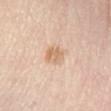Impression: The lesion was tiled from a total-body skin photograph and was not biopsied. Acquisition and patient details: The lesion is on the leg. The patient is a female in their 40s. Captured under cross-polarized illumination. The lesion-visualizer software estimated an area of roughly 6 mm² and an outline eccentricity of about 0.6 (0 = round, 1 = elongated). And it measured a classifier nevus-likeness of about 15/100 and a lesion-detection confidence of about 100/100. A 15 mm crop from a total-body photograph taken for skin-cancer surveillance.